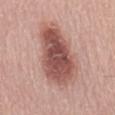notes: no biopsy performed (imaged during a skin exam)
patient: male, aged around 60
anatomic site: the mid back
image source: ~15 mm tile from a whole-body skin photo